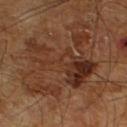Clinical impression:
Captured during whole-body skin photography for melanoma surveillance; the lesion was not biopsied.
Background:
The lesion-visualizer software estimated border irregularity of about 9 on a 0–10 scale, a color-variation rating of about 7/10, and radial color variation of about 2. The software also gave an automated nevus-likeness rating near 0 out of 100 and a lesion-detection confidence of about 100/100. A lesion tile, about 15 mm wide, cut from a 3D total-body photograph. Located on the left lower leg. Longest diameter approximately 8.5 mm. A male patient, aged 63 to 67.